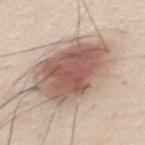Assessment:
Captured during whole-body skin photography for melanoma surveillance; the lesion was not biopsied.
Background:
Located on the mid back. The subject is a male roughly 25 years of age. A close-up tile cropped from a whole-body skin photograph, about 15 mm across.